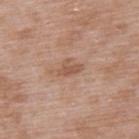Notes:
– workup: imaged on a skin check; not biopsied
– acquisition: ~15 mm crop, total-body skin-cancer survey
– site: the upper back
– tile lighting: white-light illumination
– patient: male, aged 48 to 52